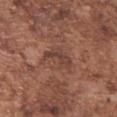No biopsy was performed on this lesion — it was imaged during a full skin examination and was not determined to be concerning.
The tile uses white-light illumination.
A close-up tile cropped from a whole-body skin photograph, about 15 mm across.
Automated image analysis of the tile measured an area of roughly 6.5 mm² and two-axis asymmetry of about 0.5. It also reported an average lesion color of about L≈42 a*≈20 b*≈25 (CIELAB) and about 7 CIELAB-L* units darker than the surrounding skin.
The patient is a male about 75 years old.
On the left upper arm.